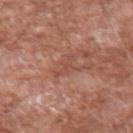Impression: Captured during whole-body skin photography for melanoma surveillance; the lesion was not biopsied. Context: This is a white-light tile. The lesion is located on the left upper arm. A male subject about 60 years old. A lesion tile, about 15 mm wide, cut from a 3D total-body photograph. The total-body-photography lesion software estimated a border-irregularity index near 7.5/10, internal color variation of about 0 on a 0–10 scale, and peripheral color asymmetry of about 0. The software also gave lesion-presence confidence of about 95/100. The lesion's longest dimension is about 3.5 mm.